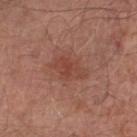{"biopsy_status": "not biopsied; imaged during a skin examination", "image": {"source": "total-body photography crop", "field_of_view_mm": 15}, "patient": {"sex": "male", "age_approx": 65}, "site": "left lower leg"}